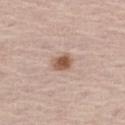biopsy_status: not biopsied; imaged during a skin examination
lighting: white-light
site: abdomen
lesion_size:
  long_diameter_mm_approx: 2.5
image:
  source: total-body photography crop
  field_of_view_mm: 15
patient:
  sex: male
  age_approx: 65
automated_metrics:
  area_mm2_approx: 4.5
  eccentricity: 0.75
  shape_asymmetry: 0.25
  cielab_L: 56
  cielab_a: 20
  cielab_b: 28
  vs_skin_darker_L: 13.0
  vs_skin_contrast_norm: 9.5
  border_irregularity_0_10: 2.5
  color_variation_0_10: 4.5
  peripheral_color_asymmetry: 1.0
  lesion_detection_confidence_0_100: 100